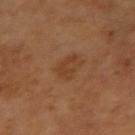Imaged with cross-polarized lighting. Located on the left arm. A male patient, approximately 65 years of age. Cropped from a total-body skin-imaging series; the visible field is about 15 mm. An algorithmic analysis of the crop reported a footprint of about 3.5 mm². It also reported a border-irregularity rating of about 7/10 and a within-lesion color-variation index near 0/10. The software also gave a classifier nevus-likeness of about 10/100 and lesion-presence confidence of about 100/100.